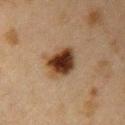follow-up — catalogued during a skin exam; not biopsied
subject — female, aged approximately 40
body site — the right upper arm
diameter — ~4 mm (longest diameter)
illumination — cross-polarized
acquisition — ~15 mm tile from a whole-body skin photo